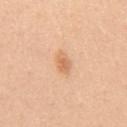{"biopsy_status": "not biopsied; imaged during a skin examination", "image": {"source": "total-body photography crop", "field_of_view_mm": 15}, "site": "abdomen", "patient": {"sex": "male", "age_approx": 50}}